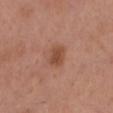Q: Is there a histopathology result?
A: no biopsy performed (imaged during a skin exam)
Q: Who is the patient?
A: male, about 55 years old
Q: Where on the body is the lesion?
A: the head or neck
Q: What lighting was used for the tile?
A: white-light illumination
Q: What kind of image is this?
A: ~15 mm crop, total-body skin-cancer survey
Q: Lesion size?
A: about 3 mm